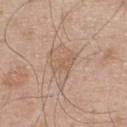  biopsy_status: not biopsied; imaged during a skin examination
  patient:
    sex: male
    age_approx: 55
  image:
    source: total-body photography crop
    field_of_view_mm: 15
  lesion_size:
    long_diameter_mm_approx: 3.0
  lighting: white-light
  site: upper back